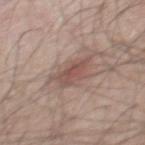follow-up = imaged on a skin check; not biopsied
image source = ~15 mm tile from a whole-body skin photo
patient = male, approximately 50 years of age
site = the mid back